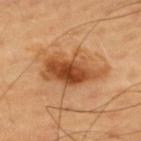Q: Was a biopsy performed?
A: imaged on a skin check; not biopsied
Q: Automated lesion metrics?
A: an average lesion color of about L≈49 a*≈25 b*≈40 (CIELAB), roughly 14 lightness units darker than nearby skin, and a normalized lesion–skin contrast near 9.5; border irregularity of about 4.5 on a 0–10 scale, internal color variation of about 8 on a 0–10 scale, and radial color variation of about 3; a nevus-likeness score of about 70/100 and a detector confidence of about 100 out of 100 that the crop contains a lesion
Q: What is the anatomic site?
A: the mid back
Q: What is the lesion's diameter?
A: about 7 mm
Q: What kind of image is this?
A: ~15 mm crop, total-body skin-cancer survey
Q: Illumination type?
A: cross-polarized illumination
Q: Patient demographics?
A: male, aged 63 to 67